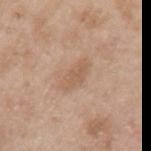follow-up — total-body-photography surveillance lesion; no biopsy
location — the arm
image-analysis metrics — an area of roughly 6.5 mm² and a shape eccentricity near 0.85; an average lesion color of about L≈59 a*≈18 b*≈31 (CIELAB), a lesion–skin lightness drop of about 7, and a lesion-to-skin contrast of about 5 (normalized; higher = more distinct)
tile lighting — white-light illumination
size — ~4 mm (longest diameter)
patient — male, in their 50s
acquisition — ~15 mm crop, total-body skin-cancer survey